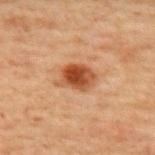| feature | finding |
|---|---|
| workup | imaged on a skin check; not biopsied |
| anatomic site | the mid back |
| imaging modality | 15 mm crop, total-body photography |
| illumination | cross-polarized illumination |
| patient | male, approximately 70 years of age |
| lesion diameter | ~3.5 mm (longest diameter) |